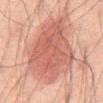The lesion was photographed on a routine skin check and not biopsied; there is no pathology result.
Automated image analysis of the tile measured an area of roughly 44 mm², an outline eccentricity of about 0.75 (0 = round, 1 = elongated), and a shape-asymmetry score of about 0.25 (0 = symmetric).
A male subject aged around 65.
The lesion is located on the mid back.
A 15 mm close-up tile from a total-body photography series done for melanoma screening.
About 9.5 mm across.